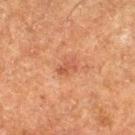Clinical impression: The lesion was tiled from a total-body skin photograph and was not biopsied. Background: Automated tile analysis of the lesion measured a lesion area of about 3.5 mm², a shape eccentricity near 0.75, and two-axis asymmetry of about 0.35. It also reported a lesion color around L≈44 a*≈24 b*≈30 in CIELAB, about 7 CIELAB-L* units darker than the surrounding skin, and a lesion-to-skin contrast of about 5.5 (normalized; higher = more distinct). A male patient aged 73–77. The tile uses cross-polarized illumination. On the right thigh. This image is a 15 mm lesion crop taken from a total-body photograph. Measured at roughly 2.5 mm in maximum diameter.